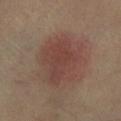| field | value |
|---|---|
| follow-up | imaged on a skin check; not biopsied |
| size | ≈6.5 mm |
| illumination | cross-polarized illumination |
| subject | in their 60s |
| body site | the leg |
| automated lesion analysis | a lesion area of about 24 mm², an outline eccentricity of about 0.5 (0 = round, 1 = elongated), and a symmetry-axis asymmetry near 0.25; a lesion color around L≈41 a*≈19 b*≈23 in CIELAB and a normalized lesion–skin contrast near 6 |
| image source | ~15 mm tile from a whole-body skin photo |